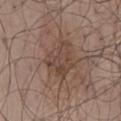  patient:
    sex: male
    age_approx: 50
  image:
    source: total-body photography crop
    field_of_view_mm: 15
  lighting: white-light
  site: mid back
  lesion_size:
    long_diameter_mm_approx: 4.5